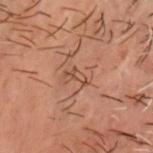Q: Was a biopsy performed?
A: no biopsy performed (imaged during a skin exam)
Q: What is the imaging modality?
A: total-body-photography crop, ~15 mm field of view
Q: What are the patient's age and sex?
A: male, about 50 years old
Q: What is the anatomic site?
A: the head or neck
Q: What lighting was used for the tile?
A: cross-polarized illumination